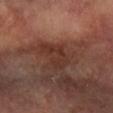tile lighting = cross-polarized illumination | patient = female, aged approximately 65 | lesion size = ~4 mm (longest diameter) | body site = the left arm | image-analysis metrics = an automated nevus-likeness rating near 0 out of 100 and a detector confidence of about 90 out of 100 that the crop contains a lesion | image source = 15 mm crop, total-body photography.